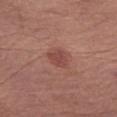Imaged during a routine full-body skin examination; the lesion was not biopsied and no histopathology is available. The tile uses white-light illumination. From the abdomen. A male patient roughly 80 years of age. A lesion tile, about 15 mm wide, cut from a 3D total-body photograph. The lesion's longest dimension is about 3 mm.